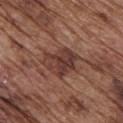<lesion>
  <biopsy_status>not biopsied; imaged during a skin examination</biopsy_status>
  <site>chest</site>
  <patient>
    <sex>male</sex>
    <age_approx>75</age_approx>
  </patient>
  <image>
    <source>total-body photography crop</source>
    <field_of_view_mm>15</field_of_view_mm>
  </image>
  <lesion_size>
    <long_diameter_mm_approx>4.5</long_diameter_mm_approx>
  </lesion_size>
  <lighting>white-light</lighting>
  <automated_metrics>
    <border_irregularity_0_10>3.0</border_irregularity_0_10>
    <color_variation_0_10>5.0</color_variation_0_10>
    <peripheral_color_asymmetry>2.0</peripheral_color_asymmetry>
    <nevus_likeness_0_100>30</nevus_likeness_0_100>
  </automated_metrics>
</lesion>